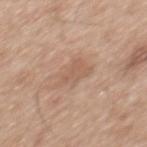notes: catalogued during a skin exam; not biopsied
diameter: ≈4 mm
anatomic site: the mid back
automated metrics: a footprint of about 7.5 mm² and a shape-asymmetry score of about 0.3 (0 = symmetric); a mean CIELAB color near L≈58 a*≈18 b*≈29 and roughly 7 lightness units darker than nearby skin; a nevus-likeness score of about 0/100 and lesion-presence confidence of about 100/100
patient: male, approximately 60 years of age
image: 15 mm crop, total-body photography